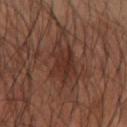Notes:
* biopsy status — catalogued during a skin exam; not biopsied
* automated lesion analysis — a lesion color around L≈31 a*≈20 b*≈24 in CIELAB, roughly 7 lightness units darker than nearby skin, and a normalized lesion–skin contrast near 7; border irregularity of about 5 on a 0–10 scale
* illumination — cross-polarized
* subject — male, aged approximately 50
* image source — ~15 mm tile from a whole-body skin photo
* size — about 4.5 mm
* site — the left arm